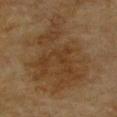<case>
<image>
  <source>total-body photography crop</source>
  <field_of_view_mm>15</field_of_view_mm>
</image>
<patient>
  <sex>male</sex>
  <age_approx>85+</age_approx>
</patient>
<site>chest</site>
</case>